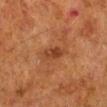Imaged during a routine full-body skin examination; the lesion was not biopsied and no histopathology is available. The subject is a male roughly 80 years of age. The lesion is located on the left lower leg. Automated tile analysis of the lesion measured an average lesion color of about L≈32 a*≈21 b*≈29 (CIELAB), a lesion–skin lightness drop of about 7, and a lesion-to-skin contrast of about 7 (normalized; higher = more distinct). And it measured a border-irregularity rating of about 2/10, a within-lesion color-variation index near 3/10, and a peripheral color-asymmetry measure near 1. The software also gave a lesion-detection confidence of about 100/100. Longest diameter approximately 3.5 mm. This is a cross-polarized tile. A 15 mm close-up extracted from a 3D total-body photography capture.